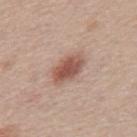<lesion>
  <biopsy_status>not biopsied; imaged during a skin examination</biopsy_status>
  <lesion_size>
    <long_diameter_mm_approx>4.5</long_diameter_mm_approx>
  </lesion_size>
  <site>mid back</site>
  <lighting>white-light</lighting>
  <image>
    <source>total-body photography crop</source>
    <field_of_view_mm>15</field_of_view_mm>
  </image>
  <patient>
    <sex>male</sex>
    <age_approx>45</age_approx>
  </patient>
</lesion>